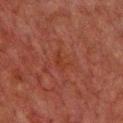lesion size=≈3 mm
body site=the chest
image source=~15 mm tile from a whole-body skin photo
subject=male, aged approximately 60